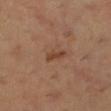Findings:
* subject · female, roughly 30 years of age
* body site · the left lower leg
* acquisition · 15 mm crop, total-body photography
* tile lighting · cross-polarized illumination